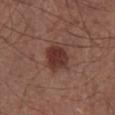Impression: Captured during whole-body skin photography for melanoma surveillance; the lesion was not biopsied. Context: The lesion is located on the abdomen. A male patient roughly 55 years of age. Cropped from a total-body skin-imaging series; the visible field is about 15 mm.